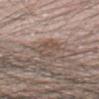<lesion>
<biopsy_status>not biopsied; imaged during a skin examination</biopsy_status>
<image>
  <source>total-body photography crop</source>
  <field_of_view_mm>15</field_of_view_mm>
</image>
<patient>
  <sex>male</sex>
  <age_approx>70</age_approx>
</patient>
<site>left forearm</site>
<lesion_size>
  <long_diameter_mm_approx>4.0</long_diameter_mm_approx>
</lesion_size>
<automated_metrics>
  <border_irregularity_0_10>4.0</border_irregularity_0_10>
  <peripheral_color_asymmetry>1.0</peripheral_color_asymmetry>
  <lesion_detection_confidence_0_100>55</lesion_detection_confidence_0_100>
</automated_metrics>
</lesion>